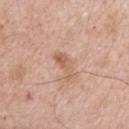No biopsy was performed on this lesion — it was imaged during a full skin examination and was not determined to be concerning. The subject is a male approximately 65 years of age. Imaged with white-light lighting. The lesion is located on the right upper arm. A 15 mm close-up tile from a total-body photography series done for melanoma screening.